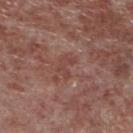workup: catalogued during a skin exam; not biopsied | TBP lesion metrics: a shape eccentricity near 0.75 and two-axis asymmetry of about 0.5; a border-irregularity rating of about 6/10, a within-lesion color-variation index near 0/10, and radial color variation of about 0; a nevus-likeness score of about 0/100 and lesion-presence confidence of about 100/100 | anatomic site: the leg | image source: ~15 mm crop, total-body skin-cancer survey | subject: male, aged 53 to 57 | lesion size: ≈2.5 mm | lighting: white-light.